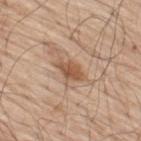workup=imaged on a skin check; not biopsied | patient=male, roughly 70 years of age | lesion size=~3.5 mm (longest diameter) | lighting=white-light illumination | anatomic site=the back | image=~15 mm tile from a whole-body skin photo | automated lesion analysis=an area of roughly 5 mm², a shape eccentricity near 0.85, and two-axis asymmetry of about 0.25; a lesion color around L≈54 a*≈20 b*≈32 in CIELAB, roughly 11 lightness units darker than nearby skin, and a normalized border contrast of about 8; a border-irregularity rating of about 2.5/10.